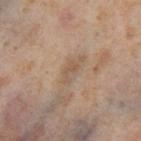Clinical impression: The lesion was photographed on a routine skin check and not biopsied; there is no pathology result. Acquisition and patient details: A female subject, approximately 55 years of age. The lesion is on the right thigh. The tile uses cross-polarized illumination. A 15 mm close-up extracted from a 3D total-body photography capture. Longest diameter approximately 3 mm. The lesion-visualizer software estimated a lesion color around L≈55 a*≈15 b*≈30 in CIELAB, a lesion–skin lightness drop of about 7, and a normalized border contrast of about 5.